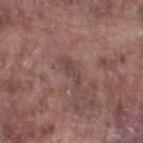Q: Is there a histopathology result?
A: catalogued during a skin exam; not biopsied
Q: What is the lesion's diameter?
A: ≈3 mm
Q: Illumination type?
A: white-light
Q: What is the imaging modality?
A: ~15 mm crop, total-body skin-cancer survey
Q: Who is the patient?
A: male, in their mid-70s
Q: What did automated image analysis measure?
A: a lesion area of about 3.5 mm² and two-axis asymmetry of about 0.4; a lesion color around L≈43 a*≈17 b*≈19 in CIELAB, about 7 CIELAB-L* units darker than the surrounding skin, and a normalized border contrast of about 5.5; a border-irregularity rating of about 5/10 and internal color variation of about 0.5 on a 0–10 scale
Q: What is the anatomic site?
A: the leg